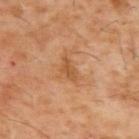<lesion>
<biopsy_status>not biopsied; imaged during a skin examination</biopsy_status>
<lesion_size>
  <long_diameter_mm_approx>3.0</long_diameter_mm_approx>
</lesion_size>
<image>
  <source>total-body photography crop</source>
  <field_of_view_mm>15</field_of_view_mm>
</image>
<patient>
  <sex>male</sex>
  <age_approx>60</age_approx>
</patient>
<lighting>cross-polarized</lighting>
<automated_metrics>
  <area_mm2_approx>3.5</area_mm2_approx>
  <shape_asymmetry>0.4</shape_asymmetry>
  <cielab_L>49</cielab_L>
  <cielab_a>21</cielab_a>
  <cielab_b>37</cielab_b>
  <vs_skin_darker_L>8.0</vs_skin_darker_L>
  <vs_skin_contrast_norm>6.0</vs_skin_contrast_norm>
</automated_metrics>
<site>upper back</site>
</lesion>